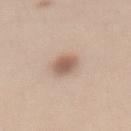{
  "biopsy_status": "not biopsied; imaged during a skin examination",
  "patient": {
    "sex": "female",
    "age_approx": 40
  },
  "lesion_size": {
    "long_diameter_mm_approx": 3.5
  },
  "automated_metrics": {
    "vs_skin_darker_L": 12.0,
    "color_variation_0_10": 3.0
  },
  "site": "abdomen",
  "image": {
    "source": "total-body photography crop",
    "field_of_view_mm": 15
  },
  "lighting": "white-light"
}